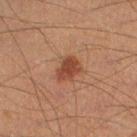Captured during whole-body skin photography for melanoma surveillance; the lesion was not biopsied. This is a cross-polarized tile. A male subject about 40 years old. Located on the left lower leg. This image is a 15 mm lesion crop taken from a total-body photograph. Longest diameter approximately 3 mm.